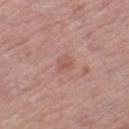The lesion was tiled from a total-body skin photograph and was not biopsied. A roughly 15 mm field-of-view crop from a total-body skin photograph. The lesion is on the leg. A female subject, aged around 55. This is a white-light tile. Approximately 2.5 mm at its widest. Automated image analysis of the tile measured a lesion color around L≈55 a*≈22 b*≈26 in CIELAB, a lesion–skin lightness drop of about 6, and a normalized border contrast of about 5.